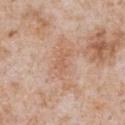Assessment: Imaged during a routine full-body skin examination; the lesion was not biopsied and no histopathology is available. Image and clinical context: A 15 mm close-up tile from a total-body photography series done for melanoma screening. A male patient, aged approximately 65. From the chest. Approximately 2.5 mm at its widest. The total-body-photography lesion software estimated a mean CIELAB color near L≈60 a*≈21 b*≈32, about 6 CIELAB-L* units darker than the surrounding skin, and a lesion-to-skin contrast of about 4.5 (normalized; higher = more distinct). The analysis additionally found a lesion-detection confidence of about 100/100. This is a white-light tile.